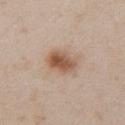Q: Was a biopsy performed?
A: no biopsy performed (imaged during a skin exam)
Q: Illumination type?
A: white-light illumination
Q: What is the anatomic site?
A: the chest
Q: How large is the lesion?
A: ≈4 mm
Q: How was this image acquired?
A: total-body-photography crop, ~15 mm field of view
Q: Who is the patient?
A: female, aged around 30